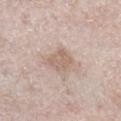Q: Was a biopsy performed?
A: total-body-photography surveillance lesion; no biopsy
Q: Lesion size?
A: about 3 mm
Q: How was this image acquired?
A: ~15 mm crop, total-body skin-cancer survey
Q: Lesion location?
A: the leg
Q: Automated lesion metrics?
A: an area of roughly 6.5 mm², a shape eccentricity near 0.7, and a shape-asymmetry score of about 0.2 (0 = symmetric); a lesion–skin lightness drop of about 9 and a lesion-to-skin contrast of about 6 (normalized; higher = more distinct); a border-irregularity index near 2/10, a color-variation rating of about 2/10, and a peripheral color-asymmetry measure near 0.5
Q: Patient demographics?
A: male, roughly 60 years of age
Q: How was the tile lit?
A: white-light illumination